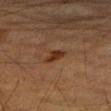{
  "biopsy_status": "not biopsied; imaged during a skin examination",
  "image": {
    "source": "total-body photography crop",
    "field_of_view_mm": 15
  },
  "patient": {
    "sex": "male",
    "age_approx": "85+"
  },
  "site": "right upper arm"
}